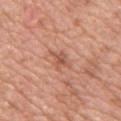{"biopsy_status": "not biopsied; imaged during a skin examination", "patient": {"sex": "female", "age_approx": 45}, "lesion_size": {"long_diameter_mm_approx": 3.5}, "image": {"source": "total-body photography crop", "field_of_view_mm": 15}, "lighting": "white-light", "site": "arm"}